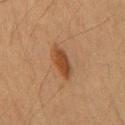Assessment:
Part of a total-body skin-imaging series; this lesion was reviewed on a skin check and was not flagged for biopsy.
Acquisition and patient details:
A male subject approximately 65 years of age. Longest diameter approximately 5 mm. Cropped from a total-body skin-imaging series; the visible field is about 15 mm. The lesion is on the chest. The total-body-photography lesion software estimated a footprint of about 7.5 mm², an outline eccentricity of about 0.95 (0 = round, 1 = elongated), and a shape-asymmetry score of about 0.25 (0 = symmetric). It also reported internal color variation of about 2.5 on a 0–10 scale and a peripheral color-asymmetry measure near 1. Captured under cross-polarized illumination.